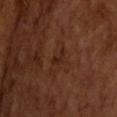Findings:
– notes — imaged on a skin check; not biopsied
– image source — ~15 mm tile from a whole-body skin photo
– subject — male, aged around 65
– lighting — cross-polarized